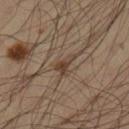Recorded during total-body skin imaging; not selected for excision or biopsy. Located on the left lower leg. The subject is a male aged 33 to 37. Cropped from a whole-body photographic skin survey; the tile spans about 15 mm. Measured at roughly 3 mm in maximum diameter.